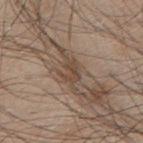Q: Was a biopsy performed?
A: imaged on a skin check; not biopsied
Q: What kind of image is this?
A: 15 mm crop, total-body photography
Q: Lesion location?
A: the back
Q: Who is the patient?
A: male, approximately 45 years of age